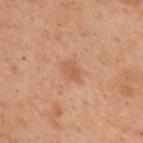biopsy_status: not biopsied; imaged during a skin examination
image:
  source: total-body photography crop
  field_of_view_mm: 15
lesion_size:
  long_diameter_mm_approx: 2.5
automated_metrics:
  area_mm2_approx: 3.5
  eccentricity: 0.7
  shape_asymmetry: 0.25
  cielab_L: 47
  cielab_a: 20
  cielab_b: 30
  vs_skin_darker_L: 6.0
  border_irregularity_0_10: 2.0
  color_variation_0_10: 2.0
  peripheral_color_asymmetry: 0.5
site: upper back
patient:
  sex: female
  age_approx: 55